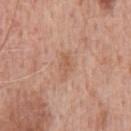Assessment:
Part of a total-body skin-imaging series; this lesion was reviewed on a skin check and was not flagged for biopsy.
Acquisition and patient details:
The lesion is located on the chest. This is a white-light tile. A male patient aged 58 to 62. A lesion tile, about 15 mm wide, cut from a 3D total-body photograph.